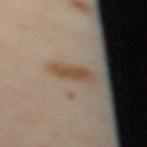Clinical impression:
The lesion was tiled from a total-body skin photograph and was not biopsied.
Clinical summary:
The lesion is located on the upper back. The lesion-visualizer software estimated an area of roughly 5 mm², an outline eccentricity of about 0.9 (0 = round, 1 = elongated), and two-axis asymmetry of about 0.25. The software also gave an average lesion color of about L≈47 a*≈15 b*≈28 (CIELAB), roughly 7 lightness units darker than nearby skin, and a lesion-to-skin contrast of about 8 (normalized; higher = more distinct). A female subject aged approximately 40. Captured under cross-polarized illumination. A 15 mm crop from a total-body photograph taken for skin-cancer surveillance. Measured at roughly 3.5 mm in maximum diameter.